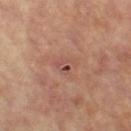Recorded during total-body skin imaging; not selected for excision or biopsy. Imaged with cross-polarized lighting. A female subject aged 63 to 67. The lesion is located on the leg. A 15 mm close-up tile from a total-body photography series done for melanoma screening.